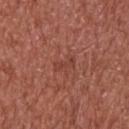| field | value |
|---|---|
| workup | total-body-photography surveillance lesion; no biopsy |
| site | the head or neck |
| patient | male, aged around 65 |
| illumination | white-light illumination |
| lesion size | about 2.5 mm |
| acquisition | 15 mm crop, total-body photography |
| image-analysis metrics | a border-irregularity index near 4.5/10, a within-lesion color-variation index near 0/10, and peripheral color asymmetry of about 0; a classifier nevus-likeness of about 0/100 and lesion-presence confidence of about 100/100 |